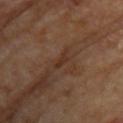The lesion was photographed on a routine skin check and not biopsied; there is no pathology result.
A 15 mm close-up tile from a total-body photography series done for melanoma screening.
A female patient approximately 65 years of age.
The lesion is located on the upper back.